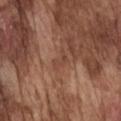The lesion was tiled from a total-body skin photograph and was not biopsied. A male patient aged around 75. On the front of the torso. A roughly 15 mm field-of-view crop from a total-body skin photograph.